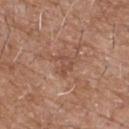<lesion>
  <biopsy_status>not biopsied; imaged during a skin examination</biopsy_status>
  <lesion_size>
    <long_diameter_mm_approx>2.5</long_diameter_mm_approx>
  </lesion_size>
  <image>
    <source>total-body photography crop</source>
    <field_of_view_mm>15</field_of_view_mm>
  </image>
  <patient>
    <sex>male</sex>
    <age_approx>60</age_approx>
  </patient>
  <site>chest</site>
</lesion>